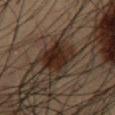{"patient": {"sex": "male", "age_approx": 55}, "site": "abdomen", "image": {"source": "total-body photography crop", "field_of_view_mm": 15}}